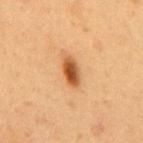Q: Is there a histopathology result?
A: catalogued during a skin exam; not biopsied
Q: Illumination type?
A: cross-polarized illumination
Q: What is the anatomic site?
A: the mid back
Q: How was this image acquired?
A: ~15 mm crop, total-body skin-cancer survey
Q: Lesion size?
A: about 4 mm
Q: Patient demographics?
A: male, aged 53–57